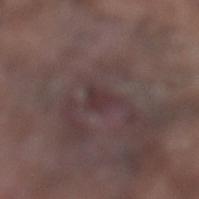  biopsy_status: not biopsied; imaged during a skin examination